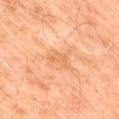- notes: total-body-photography surveillance lesion; no biopsy
- size: ≈3 mm
- body site: the front of the torso
- image source: ~15 mm crop, total-body skin-cancer survey
- tile lighting: cross-polarized illumination
- image-analysis metrics: an eccentricity of roughly 0.8 and two-axis asymmetry of about 0.45; a lesion color around L≈65 a*≈25 b*≈41 in CIELAB and a normalized border contrast of about 4.5; a border-irregularity rating of about 4.5/10, internal color variation of about 1.5 on a 0–10 scale, and a peripheral color-asymmetry measure near 0.5
- subject: male, aged around 60